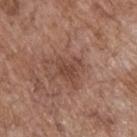Assessment: Captured during whole-body skin photography for melanoma surveillance; the lesion was not biopsied. Acquisition and patient details: A male subject aged around 70. On the upper back. This image is a 15 mm lesion crop taken from a total-body photograph. Captured under white-light illumination. The lesion's longest dimension is about 3 mm.